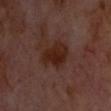follow-up: catalogued during a skin exam; not biopsied | site: the head or neck | image source: ~15 mm crop, total-body skin-cancer survey | illumination: cross-polarized illumination | patient: aged approximately 65.